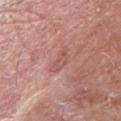Acquisition and patient details:
The subject is a male roughly 80 years of age. The lesion is on the right upper arm. Approximately 3 mm at its widest. A roughly 15 mm field-of-view crop from a total-body skin photograph. The total-body-photography lesion software estimated a classifier nevus-likeness of about 0/100 and a detector confidence of about 100 out of 100 that the crop contains a lesion.